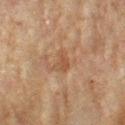workup — no biopsy performed (imaged during a skin exam) | subject — female, aged around 80 | image source — ~15 mm tile from a whole-body skin photo | anatomic site — the left forearm.